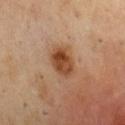{
  "biopsy_status": "not biopsied; imaged during a skin examination",
  "image": {
    "source": "total-body photography crop",
    "field_of_view_mm": 15
  },
  "lesion_size": {
    "long_diameter_mm_approx": 4.0
  },
  "patient": {
    "sex": "male",
    "age_approx": 70
  },
  "site": "chest"
}